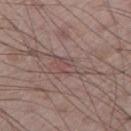biopsy_status: not biopsied; imaged during a skin examination
lesion_size:
  long_diameter_mm_approx: 3.0
image:
  source: total-body photography crop
  field_of_view_mm: 15
site: right thigh
automated_metrics:
  cielab_L: 47
  cielab_a: 18
  cielab_b: 19
  vs_skin_darker_L: 6.0
  vs_skin_contrast_norm: 4.5
  lesion_detection_confidence_0_100: 65
patient:
  sex: male
  age_approx: 75
lighting: white-light